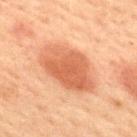{
  "biopsy_status": "not biopsied; imaged during a skin examination",
  "patient": {
    "sex": "male",
    "age_approx": 60
  },
  "site": "upper back",
  "lesion_size": {
    "long_diameter_mm_approx": 7.0
  },
  "image": {
    "source": "total-body photography crop",
    "field_of_view_mm": 15
  },
  "automated_metrics": {
    "area_mm2_approx": 23.0,
    "eccentricity": 0.8,
    "shape_asymmetry": 0.15,
    "cielab_L": 52,
    "cielab_a": 24,
    "cielab_b": 33,
    "vs_skin_darker_L": 11.0,
    "vs_skin_contrast_norm": 7.5,
    "lesion_detection_confidence_0_100": 100
  },
  "lighting": "cross-polarized"
}